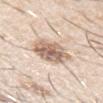Q: Patient demographics?
A: male, aged around 30
Q: What kind of image is this?
A: 15 mm crop, total-body photography
Q: Lesion location?
A: the left upper arm
Q: How large is the lesion?
A: about 4.5 mm
Q: What lighting was used for the tile?
A: white-light illumination